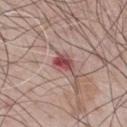{"biopsy_status": "not biopsied; imaged during a skin examination", "patient": {"sex": "male", "age_approx": 65}, "image": {"source": "total-body photography crop", "field_of_view_mm": 15}, "site": "front of the torso"}